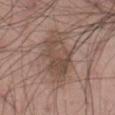<case>
<biopsy_status>not biopsied; imaged during a skin examination</biopsy_status>
<patient>
  <sex>male</sex>
  <age_approx>55</age_approx>
</patient>
<image>
  <source>total-body photography crop</source>
  <field_of_view_mm>15</field_of_view_mm>
</image>
<lesion_size>
  <long_diameter_mm_approx>5.0</long_diameter_mm_approx>
</lesion_size>
<site>abdomen</site>
</case>